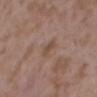{
  "biopsy_status": "not biopsied; imaged during a skin examination",
  "patient": {
    "sex": "female",
    "age_approx": 35
  },
  "lighting": "white-light",
  "lesion_size": {
    "long_diameter_mm_approx": 2.5
  },
  "automated_metrics": {
    "area_mm2_approx": 3.5,
    "shape_asymmetry": 0.3,
    "color_variation_0_10": 1.5,
    "peripheral_color_asymmetry": 0.5
  },
  "site": "back",
  "image": {
    "source": "total-body photography crop",
    "field_of_view_mm": 15
  }
}